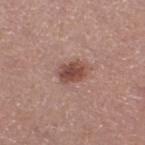Findings:
- notes: no biopsy performed (imaged during a skin exam)
- acquisition: ~15 mm tile from a whole-body skin photo
- anatomic site: the right thigh
- tile lighting: white-light
- patient: female, aged 48–52
- lesion diameter: about 3.5 mm